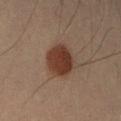Part of a total-body skin-imaging series; this lesion was reviewed on a skin check and was not flagged for biopsy.
Located on the right forearm.
Captured under cross-polarized illumination.
Automated tile analysis of the lesion measured border irregularity of about 1 on a 0–10 scale and a color-variation rating of about 3/10. The analysis additionally found a detector confidence of about 100 out of 100 that the crop contains a lesion.
Approximately 4 mm at its widest.
A 15 mm close-up tile from a total-body photography series done for melanoma screening.
A male subject, in their 40s.